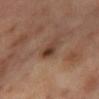Clinical impression: The lesion was photographed on a routine skin check and not biopsied; there is no pathology result. Context: Cropped from a whole-body photographic skin survey; the tile spans about 15 mm. A female subject, roughly 55 years of age. Measured at roughly 2.5 mm in maximum diameter. The lesion is located on the right thigh.